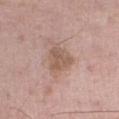Assessment:
Recorded during total-body skin imaging; not selected for excision or biopsy.
Acquisition and patient details:
The lesion's longest dimension is about 4 mm. A lesion tile, about 15 mm wide, cut from a 3D total-body photograph. The tile uses white-light illumination. An algorithmic analysis of the crop reported a lesion area of about 9 mm² and a symmetry-axis asymmetry near 0.3. The analysis additionally found an average lesion color of about L≈57 a*≈17 b*≈26 (CIELAB), about 9 CIELAB-L* units darker than the surrounding skin, and a lesion-to-skin contrast of about 6.5 (normalized; higher = more distinct). The software also gave an automated nevus-likeness rating near 5 out of 100 and a lesion-detection confidence of about 100/100. The subject is a male aged around 75. The lesion is located on the right lower leg.